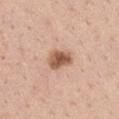This lesion was catalogued during total-body skin photography and was not selected for biopsy.
Captured under white-light illumination.
A 15 mm close-up extracted from a 3D total-body photography capture.
Measured at roughly 3 mm in maximum diameter.
Automated image analysis of the tile measured an area of roughly 6.5 mm², an outline eccentricity of about 0.7 (0 = round, 1 = elongated), and a shape-asymmetry score of about 0.15 (0 = symmetric). The analysis additionally found a classifier nevus-likeness of about 90/100.
From the left upper arm.
A male subject aged 38 to 42.